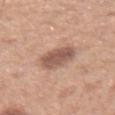The lesion was photographed on a routine skin check and not biopsied; there is no pathology result. Automated image analysis of the tile measured an area of roughly 10 mm², an outline eccentricity of about 0.55 (0 = round, 1 = elongated), and two-axis asymmetry of about 0.15. And it measured a lesion–skin lightness drop of about 13 and a lesion-to-skin contrast of about 8.5 (normalized; higher = more distinct). The software also gave border irregularity of about 1.5 on a 0–10 scale and internal color variation of about 3.5 on a 0–10 scale. And it measured a nevus-likeness score of about 15/100 and a lesion-detection confidence of about 100/100. A 15 mm crop from a total-body photograph taken for skin-cancer surveillance. About 4 mm across. The lesion is on the right forearm. Captured under white-light illumination. A female patient, about 40 years old.